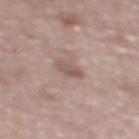Recorded during total-body skin imaging; not selected for excision or biopsy. The recorded lesion diameter is about 2.5 mm. A female patient about 65 years old. On the upper back. Cropped from a whole-body photographic skin survey; the tile spans about 15 mm.